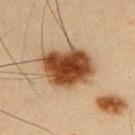{
  "image": {
    "source": "total-body photography crop",
    "field_of_view_mm": 15
  },
  "site": "upper back",
  "lighting": "cross-polarized",
  "patient": {
    "sex": "male",
    "age_approx": 35
  },
  "automated_metrics": {
    "vs_skin_darker_L": 19.0,
    "vs_skin_contrast_norm": 14.0
  },
  "lesion_size": {
    "long_diameter_mm_approx": 6.0
  }
}